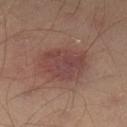Notes:
- biopsy status — no biopsy performed (imaged during a skin exam)
- site — the right leg
- patient — male, aged 53–57
- image — ~15 mm crop, total-body skin-cancer survey